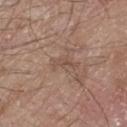Approximately 2.5 mm at its widest. The lesion is on the left thigh. A 15 mm close-up extracted from a 3D total-body photography capture. This is a white-light tile. A male subject, aged 78 to 82.